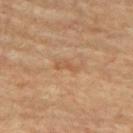Clinical impression: This lesion was catalogued during total-body skin photography and was not selected for biopsy. Clinical summary: Located on the upper back. This image is a 15 mm lesion crop taken from a total-body photograph. The lesion's longest dimension is about 2.5 mm. A female patient, aged 78 to 82.